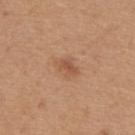Captured during whole-body skin photography for melanoma surveillance; the lesion was not biopsied.
On the upper back.
A female patient aged 53 to 57.
A 15 mm close-up tile from a total-body photography series done for melanoma screening.
Captured under white-light illumination.